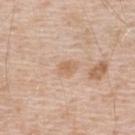Part of a total-body skin-imaging series; this lesion was reviewed on a skin check and was not flagged for biopsy.
The lesion is on the upper back.
A male patient, aged 48–52.
The recorded lesion diameter is about 2.5 mm.
The tile uses white-light illumination.
A close-up tile cropped from a whole-body skin photograph, about 15 mm across.